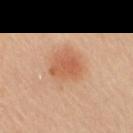{"biopsy_status": "not biopsied; imaged during a skin examination", "site": "arm", "image": {"source": "total-body photography crop", "field_of_view_mm": 15}, "patient": {"sex": "female", "age_approx": 40}, "lighting": "cross-polarized", "lesion_size": {"long_diameter_mm_approx": 4.0}}